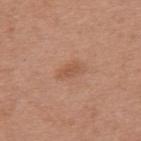The lesion was photographed on a routine skin check and not biopsied; there is no pathology result.
A 15 mm crop from a total-body photograph taken for skin-cancer surveillance.
The subject is a female roughly 35 years of age.
An algorithmic analysis of the crop reported an area of roughly 3 mm², a shape eccentricity near 0.85, and a symmetry-axis asymmetry near 0.25. The analysis additionally found a classifier nevus-likeness of about 10/100 and lesion-presence confidence of about 100/100.
On the upper back.